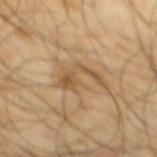biopsy_status: not biopsied; imaged during a skin examination
site: back
image:
  source: total-body photography crop
  field_of_view_mm: 15
patient:
  sex: male
  age_approx: 65
automated_metrics:
  vs_skin_darker_L: 8.0
  vs_skin_contrast_norm: 6.5
  nevus_likeness_0_100: 5
  lesion_detection_confidence_0_100: 65
lighting: cross-polarized